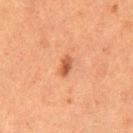Clinical impression:
Imaged during a routine full-body skin examination; the lesion was not biopsied and no histopathology is available.